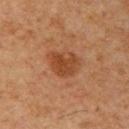Q: Was a biopsy performed?
A: total-body-photography surveillance lesion; no biopsy
Q: How large is the lesion?
A: about 4 mm
Q: Patient demographics?
A: male, aged approximately 60
Q: Automated lesion metrics?
A: an area of roughly 9 mm², a shape eccentricity near 0.6, and a shape-asymmetry score of about 0.3 (0 = symmetric); a border-irregularity index near 3.5/10 and a peripheral color-asymmetry measure near 1; a nevus-likeness score of about 80/100 and a lesion-detection confidence of about 100/100
Q: Lesion location?
A: the right upper arm
Q: How was the tile lit?
A: cross-polarized illumination
Q: What is the imaging modality?
A: 15 mm crop, total-body photography